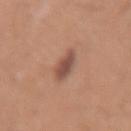lesion size = ≈3 mm; anatomic site = the right forearm; patient = female, in their mid-40s; imaging modality = total-body-photography crop, ~15 mm field of view.